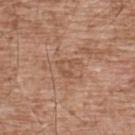notes: imaged on a skin check; not biopsied | site: the upper back | tile lighting: white-light illumination | image-analysis metrics: an outline eccentricity of about 0.5 (0 = round, 1 = elongated) and a shape-asymmetry score of about 0.5 (0 = symmetric); a mean CIELAB color near L≈52 a*≈20 b*≈31, roughly 6 lightness units darker than nearby skin, and a normalized border contrast of about 5; a border-irregularity rating of about 5/10, a within-lesion color-variation index near 3/10, and peripheral color asymmetry of about 1; an automated nevus-likeness rating near 0 out of 100 | patient: male, about 55 years old | acquisition: total-body-photography crop, ~15 mm field of view.